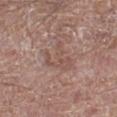{
  "biopsy_status": "not biopsied; imaged during a skin examination",
  "patient": {
    "sex": "male",
    "age_approx": 65
  },
  "lesion_size": {
    "long_diameter_mm_approx": 4.0
  },
  "automated_metrics": {
    "area_mm2_approx": 7.5,
    "eccentricity": 0.5,
    "shape_asymmetry": 0.6,
    "color_variation_0_10": 1.5,
    "peripheral_color_asymmetry": 0.5,
    "nevus_likeness_0_100": 0,
    "lesion_detection_confidence_0_100": 90
  },
  "site": "left lower leg",
  "lighting": "white-light",
  "image": {
    "source": "total-body photography crop",
    "field_of_view_mm": 15
  }
}